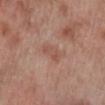{"biopsy_status": "not biopsied; imaged during a skin examination", "lighting": "white-light", "image": {"source": "total-body photography crop", "field_of_view_mm": 15}, "automated_metrics": {"lesion_detection_confidence_0_100": 100}, "lesion_size": {"long_diameter_mm_approx": 3.5}, "site": "right lower leg", "patient": {"sex": "male", "age_approx": 70}}